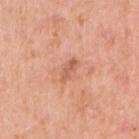Assessment:
The lesion was photographed on a routine skin check and not biopsied; there is no pathology result.
Background:
Cropped from a whole-body photographic skin survey; the tile spans about 15 mm. About 3 mm across. The tile uses white-light illumination. A female patient aged around 40. The lesion is located on the right upper arm.